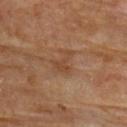subject=female, aged 78 to 82
automated metrics=an eccentricity of roughly 0.85; a border-irregularity rating of about 6.5/10, a within-lesion color-variation index near 1.5/10, and peripheral color asymmetry of about 0.5
body site=the upper back
lesion diameter=~3 mm (longest diameter)
image=15 mm crop, total-body photography
tile lighting=cross-polarized